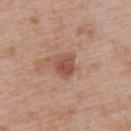notes: catalogued during a skin exam; not biopsied | diameter: ~2.5 mm (longest diameter) | body site: the upper back | patient: male, aged 53 to 57 | tile lighting: white-light illumination | image: ~15 mm crop, total-body skin-cancer survey.